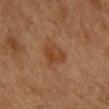<case>
<biopsy_status>not biopsied; imaged during a skin examination</biopsy_status>
<lesion_size>
  <long_diameter_mm_approx>3.5</long_diameter_mm_approx>
</lesion_size>
<site>left forearm</site>
<patient>
  <sex>female</sex>
  <age_approx>55</age_approx>
</patient>
<image>
  <source>total-body photography crop</source>
  <field_of_view_mm>15</field_of_view_mm>
</image>
<lighting>cross-polarized</lighting>
</case>